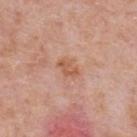Clinical impression:
This lesion was catalogued during total-body skin photography and was not selected for biopsy.
Background:
A roughly 15 mm field-of-view crop from a total-body skin photograph. The patient is a male in their mid-70s. Located on the upper back.